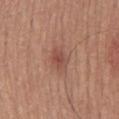<tbp_lesion>
  <biopsy_status>not biopsied; imaged during a skin examination</biopsy_status>
  <lesion_size>
    <long_diameter_mm_approx>3.5</long_diameter_mm_approx>
  </lesion_size>
  <image>
    <source>total-body photography crop</source>
    <field_of_view_mm>15</field_of_view_mm>
  </image>
  <site>back</site>
  <patient>
    <sex>male</sex>
    <age_approx>55</age_approx>
  </patient>
  <lighting>white-light</lighting>
</tbp_lesion>